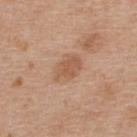biopsy status = total-body-photography surveillance lesion; no biopsy | diameter = ≈3.5 mm | body site = the upper back | patient = male, aged 63 to 67 | imaging modality = total-body-photography crop, ~15 mm field of view | lighting = white-light.